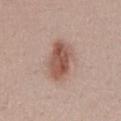<lesion>
  <biopsy_status>not biopsied; imaged during a skin examination</biopsy_status>
  <site>lower back</site>
  <patient>
    <sex>male</sex>
    <age_approx>25</age_approx>
  </patient>
  <image>
    <source>total-body photography crop</source>
    <field_of_view_mm>15</field_of_view_mm>
  </image>
  <lighting>white-light</lighting>
</lesion>